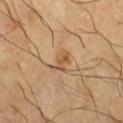Assessment: Part of a total-body skin-imaging series; this lesion was reviewed on a skin check and was not flagged for biopsy. Acquisition and patient details: The tile uses cross-polarized illumination. About 3 mm across. A male patient in their mid- to late 60s. From the right lower leg. A roughly 15 mm field-of-view crop from a total-body skin photograph.